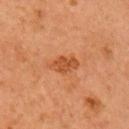<case>
<biopsy_status>not biopsied; imaged during a skin examination</biopsy_status>
<patient>
  <sex>male</sex>
  <age_approx>55</age_approx>
</patient>
<image>
  <source>total-body photography crop</source>
  <field_of_view_mm>15</field_of_view_mm>
</image>
<site>right upper arm</site>
<lesion_size>
  <long_diameter_mm_approx>3.5</long_diameter_mm_approx>
</lesion_size>
<lighting>cross-polarized</lighting>
<automated_metrics>
  <area_mm2_approx>5.5</area_mm2_approx>
  <shape_asymmetry>0.2</shape_asymmetry>
  <cielab_L>48</cielab_L>
  <cielab_a>27</cielab_a>
  <cielab_b>39</cielab_b>
  <vs_skin_darker_L>9.0</vs_skin_darker_L>
  <border_irregularity_0_10>2.0</border_irregularity_0_10>
  <color_variation_0_10>2.0</color_variation_0_10>
  <peripheral_color_asymmetry>1.0</peripheral_color_asymmetry>
  <lesion_detection_confidence_0_100>100</lesion_detection_confidence_0_100>
</automated_metrics>
</case>